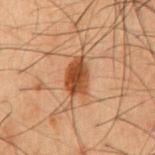Impression: This lesion was catalogued during total-body skin photography and was not selected for biopsy. Clinical summary: A male subject aged 48–52. Cropped from a total-body skin-imaging series; the visible field is about 15 mm. On the abdomen. This is a cross-polarized tile.